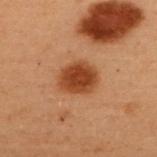Captured during whole-body skin photography for melanoma surveillance; the lesion was not biopsied. A female patient, in their 50s. This is a cross-polarized tile. From the upper back. A 15 mm crop from a total-body photograph taken for skin-cancer surveillance. The total-body-photography lesion software estimated about 12 CIELAB-L* units darker than the surrounding skin and a normalized border contrast of about 10.5. The analysis additionally found a nevus-likeness score of about 100/100 and lesion-presence confidence of about 100/100.